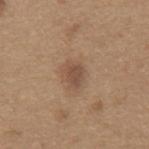biopsy status = total-body-photography surveillance lesion; no biopsy
lighting = white-light
lesion size = ≈3 mm
acquisition = ~15 mm crop, total-body skin-cancer survey
site = the mid back
automated lesion analysis = a footprint of about 4.5 mm² and two-axis asymmetry of about 0.3; a border-irregularity index near 2.5/10, internal color variation of about 2 on a 0–10 scale, and peripheral color asymmetry of about 0.5
patient = male, about 65 years old